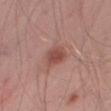Recorded during total-body skin imaging; not selected for excision or biopsy. The lesion is located on the right thigh. The recorded lesion diameter is about 3 mm. A male patient, aged around 55. A lesion tile, about 15 mm wide, cut from a 3D total-body photograph.